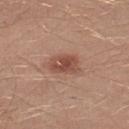Q: Is there a histopathology result?
A: no biopsy performed (imaged during a skin exam)
Q: Patient demographics?
A: male, in their 30s
Q: What did automated image analysis measure?
A: a lesion area of about 8 mm² and an eccentricity of roughly 0.7; a classifier nevus-likeness of about 80/100 and lesion-presence confidence of about 100/100
Q: Lesion size?
A: ~3.5 mm (longest diameter)
Q: Illumination type?
A: white-light illumination
Q: What kind of image is this?
A: ~15 mm crop, total-body skin-cancer survey
Q: Where on the body is the lesion?
A: the left lower leg